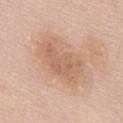Q: Was this lesion biopsied?
A: imaged on a skin check; not biopsied